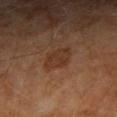{
  "biopsy_status": "not biopsied; imaged during a skin examination",
  "lesion_size": {
    "long_diameter_mm_approx": 3.0
  },
  "lighting": "cross-polarized",
  "patient": {
    "sex": "male",
    "age_approx": 55
  },
  "image": {
    "source": "total-body photography crop",
    "field_of_view_mm": 15
  },
  "automated_metrics": {
    "area_mm2_approx": 6.5,
    "eccentricity": 0.65,
    "shape_asymmetry": 0.2,
    "vs_skin_darker_L": 6.0,
    "vs_skin_contrast_norm": 6.0,
    "border_irregularity_0_10": 2.0,
    "color_variation_0_10": 2.0,
    "peripheral_color_asymmetry": 0.5
  },
  "site": "right upper arm"
}